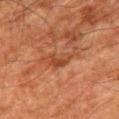Findings:
* workup · catalogued during a skin exam; not biopsied
* acquisition · 15 mm crop, total-body photography
* patient · male, roughly 80 years of age
* body site · the right thigh